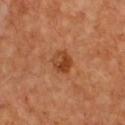{"biopsy_status": "not biopsied; imaged during a skin examination", "lighting": "cross-polarized", "image": {"source": "total-body photography crop", "field_of_view_mm": 15}, "patient": {"sex": "female", "age_approx": 50}, "lesion_size": {"long_diameter_mm_approx": 3.0}, "automated_metrics": {"cielab_L": 42, "cielab_a": 26, "cielab_b": 37, "vs_skin_darker_L": 10.0, "vs_skin_contrast_norm": 8.0}, "site": "chest"}